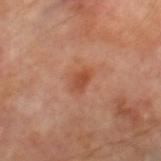  biopsy_status: not biopsied; imaged during a skin examination
  lighting: cross-polarized
  lesion_size:
    long_diameter_mm_approx: 2.5
  image:
    source: total-body photography crop
    field_of_view_mm: 15
  patient:
    sex: male
    age_approx: 70
  automated_metrics:
    area_mm2_approx: 4.0
    eccentricity: 0.75
    shape_asymmetry: 0.25
    border_irregularity_0_10: 2.5
    color_variation_0_10: 3.0
    peripheral_color_asymmetry: 1.0
  site: right thigh